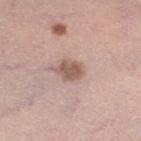Captured during whole-body skin photography for melanoma surveillance; the lesion was not biopsied. This is a white-light tile. Measured at roughly 3 mm in maximum diameter. Automated image analysis of the tile measured an eccentricity of roughly 0.6 and a symmetry-axis asymmetry near 0.2. The analysis additionally found an average lesion color of about L≈56 a*≈18 b*≈25 (CIELAB), roughly 12 lightness units darker than nearby skin, and a normalized lesion–skin contrast near 8. And it measured a border-irregularity index near 2/10 and peripheral color asymmetry of about 0.5. The software also gave a nevus-likeness score of about 75/100 and lesion-presence confidence of about 100/100. The patient is a female about 65 years old. A 15 mm close-up tile from a total-body photography series done for melanoma screening. From the left lower leg.